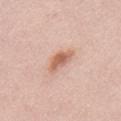Imaged during a routine full-body skin examination; the lesion was not biopsied and no histopathology is available.
The lesion is on the abdomen.
A male subject, approximately 50 years of age.
A 15 mm close-up extracted from a 3D total-body photography capture.